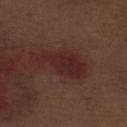Q: Was this lesion biopsied?
A: no biopsy performed (imaged during a skin exam)
Q: Lesion location?
A: the left thigh
Q: Automated lesion metrics?
A: a lesion area of about 13 mm² and a shape eccentricity near 0.9; about 7 CIELAB-L* units darker than the surrounding skin; an automated nevus-likeness rating near 30 out of 100 and a detector confidence of about 100 out of 100 that the crop contains a lesion
Q: What is the lesion's diameter?
A: about 6.5 mm
Q: Who is the patient?
A: male, approximately 70 years of age
Q: What kind of image is this?
A: ~15 mm tile from a whole-body skin photo